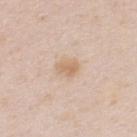<lesion>
  <biopsy_status>not biopsied; imaged during a skin examination</biopsy_status>
  <image>
    <source>total-body photography crop</source>
    <field_of_view_mm>15</field_of_view_mm>
  </image>
  <lighting>white-light</lighting>
  <site>upper back</site>
  <patient>
    <sex>male</sex>
    <age_approx>35</age_approx>
  </patient>
  <lesion_size>
    <long_diameter_mm_approx>2.5</long_diameter_mm_approx>
  </lesion_size>
</lesion>